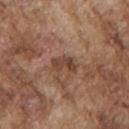{"biopsy_status": "not biopsied; imaged during a skin examination", "patient": {"sex": "male", "age_approx": 75}, "lighting": "white-light", "site": "left upper arm", "lesion_size": {"long_diameter_mm_approx": 3.0}, "image": {"source": "total-body photography crop", "field_of_view_mm": 15}}